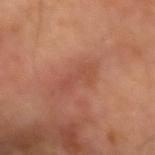The lesion was tiled from a total-body skin photograph and was not biopsied.
Located on the left forearm.
Measured at roughly 4.5 mm in maximum diameter.
A lesion tile, about 15 mm wide, cut from a 3D total-body photograph.
A male subject in their 70s.
The total-body-photography lesion software estimated an eccentricity of roughly 0.95 and a symmetry-axis asymmetry near 0.3.
The tile uses cross-polarized illumination.